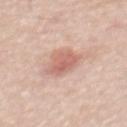Case summary:
- biopsy status · total-body-photography surveillance lesion; no biopsy
- lesion size · ~3.5 mm (longest diameter)
- image · ~15 mm crop, total-body skin-cancer survey
- image-analysis metrics · a lesion area of about 8 mm², an outline eccentricity of about 0.45 (0 = round, 1 = elongated), and a shape-asymmetry score of about 0.25 (0 = symmetric); an average lesion color of about L≈63 a*≈24 b*≈27 (CIELAB), about 10 CIELAB-L* units darker than the surrounding skin, and a normalized lesion–skin contrast near 6.5
- anatomic site · the upper back
- patient · male, roughly 60 years of age
- tile lighting · white-light illumination